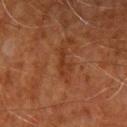Clinical impression:
Recorded during total-body skin imaging; not selected for excision or biopsy.
Context:
A male patient aged approximately 70. Located on the right upper arm. Captured under cross-polarized illumination. This image is a 15 mm lesion crop taken from a total-body photograph.